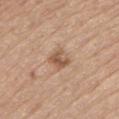* follow-up · catalogued during a skin exam; not biopsied
* patient · male, roughly 70 years of age
* illumination · white-light illumination
* imaging modality · 15 mm crop, total-body photography
* location · the lower back
* size · ~2.5 mm (longest diameter)